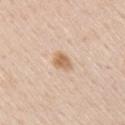This lesion was catalogued during total-body skin photography and was not selected for biopsy.
The lesion is on the right upper arm.
The patient is a male approximately 50 years of age.
The total-body-photography lesion software estimated an area of roughly 4.5 mm², a shape eccentricity near 0.6, and two-axis asymmetry of about 0.2. The analysis additionally found an average lesion color of about L≈65 a*≈18 b*≈34 (CIELAB) and a lesion-to-skin contrast of about 8 (normalized; higher = more distinct). It also reported border irregularity of about 1.5 on a 0–10 scale and a within-lesion color-variation index near 3.5/10.
Captured under white-light illumination.
A region of skin cropped from a whole-body photographic capture, roughly 15 mm wide.
The recorded lesion diameter is about 2.5 mm.